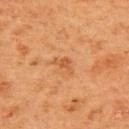workup: imaged on a skin check; not biopsied
image: 15 mm crop, total-body photography
patient: female, aged around 50
image-analysis metrics: a lesion-to-skin contrast of about 5.5 (normalized; higher = more distinct)
anatomic site: the back
illumination: cross-polarized illumination